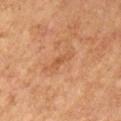Notes:
- notes: no biopsy performed (imaged during a skin exam)
- patient: female, aged around 60
- body site: the left upper arm
- image-analysis metrics: a lesion color around L≈48 a*≈23 b*≈34 in CIELAB and a normalized border contrast of about 5; a border-irregularity rating of about 4.5/10, internal color variation of about 0 on a 0–10 scale, and peripheral color asymmetry of about 0
- illumination: cross-polarized
- acquisition: ~15 mm crop, total-body skin-cancer survey
- lesion diameter: ~2.5 mm (longest diameter)